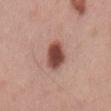Clinical impression:
Imaged during a routine full-body skin examination; the lesion was not biopsied and no histopathology is available.
Image and clinical context:
Measured at roughly 4 mm in maximum diameter. A male patient, about 50 years old. Imaged with white-light lighting. The lesion is on the mid back. Cropped from a whole-body photographic skin survey; the tile spans about 15 mm.